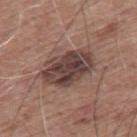Case summary:
• follow-up: imaged on a skin check; not biopsied
• illumination: white-light
• subject: male, aged approximately 60
• body site: the back
• image source: total-body-photography crop, ~15 mm field of view
• diameter: ≈6 mm